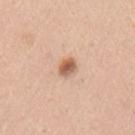Part of a total-body skin-imaging series; this lesion was reviewed on a skin check and was not flagged for biopsy. A 15 mm crop from a total-body photograph taken for skin-cancer surveillance. A male subject, aged around 50. The lesion-visualizer software estimated a lesion area of about 4 mm² and a symmetry-axis asymmetry near 0.2. The analysis additionally found an average lesion color of about L≈60 a*≈22 b*≈31 (CIELAB). The software also gave a classifier nevus-likeness of about 95/100 and a lesion-detection confidence of about 100/100. From the left upper arm. Imaged with white-light lighting.